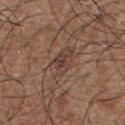{"biopsy_status": "not biopsied; imaged during a skin examination", "image": {"source": "total-body photography crop", "field_of_view_mm": 15}, "patient": {"sex": "male", "age_approx": 55}, "site": "chest", "lesion_size": {"long_diameter_mm_approx": 4.5}}